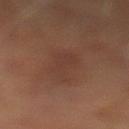Imaged during a routine full-body skin examination; the lesion was not biopsied and no histopathology is available. Measured at roughly 6.5 mm in maximum diameter. The subject is a male aged 63 to 67. This is a cross-polarized tile. An algorithmic analysis of the crop reported a lesion area of about 11 mm², a shape eccentricity near 0.95, and two-axis asymmetry of about 0.35. The lesion is located on the left lower leg. A region of skin cropped from a whole-body photographic capture, roughly 15 mm wide.